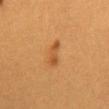{"biopsy_status": "not biopsied; imaged during a skin examination", "patient": {"sex": "female", "age_approx": 30}, "lesion_size": {"long_diameter_mm_approx": 3.0}, "image": {"source": "total-body photography crop", "field_of_view_mm": 15}, "lighting": "cross-polarized", "site": "abdomen", "automated_metrics": {"area_mm2_approx": 4.0, "eccentricity": 0.9, "vs_skin_darker_L": 9.0, "vs_skin_contrast_norm": 6.5, "border_irregularity_0_10": 4.5, "color_variation_0_10": 1.0, "nevus_likeness_0_100": 80, "lesion_detection_confidence_0_100": 100}}